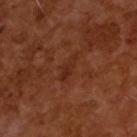<tbp_lesion>
<lighting>cross-polarized</lighting>
<automated_metrics>
  <area_mm2_approx>4.0</area_mm2_approx>
  <shape_asymmetry>0.3</shape_asymmetry>
  <cielab_L>28</cielab_L>
  <cielab_a>24</cielab_a>
  <cielab_b>29</cielab_b>
  <vs_skin_darker_L>5.0</vs_skin_darker_L>
  <vs_skin_contrast_norm>5.0</vs_skin_contrast_norm>
  <border_irregularity_0_10>4.0</border_irregularity_0_10>
  <color_variation_0_10>1.0</color_variation_0_10>
  <peripheral_color_asymmetry>0.0</peripheral_color_asymmetry>
  <nevus_likeness_0_100>0</nevus_likeness_0_100>
  <lesion_detection_confidence_0_100>100</lesion_detection_confidence_0_100>
</automated_metrics>
<lesion_size>
  <long_diameter_mm_approx>3.5</long_diameter_mm_approx>
</lesion_size>
<image>
  <source>total-body photography crop</source>
  <field_of_view_mm>15</field_of_view_mm>
</image>
<patient>
  <sex>male</sex>
  <age_approx>65</age_approx>
</patient>
</tbp_lesion>